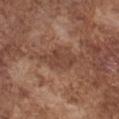An algorithmic analysis of the crop reported a lesion area of about 7.5 mm², an eccentricity of roughly 0.85, and two-axis asymmetry of about 0.35. The lesion is on the chest. Measured at roughly 4.5 mm in maximum diameter. This is a white-light tile. A roughly 15 mm field-of-view crop from a total-body skin photograph. A male patient, approximately 75 years of age.